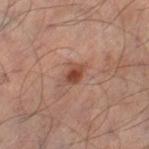No biopsy was performed on this lesion — it was imaged during a full skin examination and was not determined to be concerning.
A male subject, about 50 years old.
A 15 mm close-up extracted from a 3D total-body photography capture.
The lesion-visualizer software estimated a lesion area of about 4 mm², an eccentricity of roughly 0.7, and a shape-asymmetry score of about 0.2 (0 = symmetric). And it measured a within-lesion color-variation index near 3.5/10. The analysis additionally found a classifier nevus-likeness of about 80/100 and lesion-presence confidence of about 100/100.
Captured under cross-polarized illumination.
Longest diameter approximately 2.5 mm.
The lesion is on the left leg.